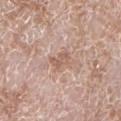{"biopsy_status": "not biopsied; imaged during a skin examination", "site": "left lower leg", "lighting": "white-light", "patient": {"sex": "male", "age_approx": 60}, "lesion_size": {"long_diameter_mm_approx": 3.0}, "image": {"source": "total-body photography crop", "field_of_view_mm": 15}}